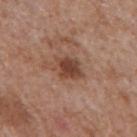{
  "biopsy_status": "not biopsied; imaged during a skin examination",
  "patient": {
    "sex": "male",
    "age_approx": 65
  },
  "automated_metrics": {
    "cielab_L": 44,
    "cielab_a": 21,
    "cielab_b": 28,
    "vs_skin_darker_L": 11.0,
    "vs_skin_contrast_norm": 8.5,
    "border_irregularity_0_10": 3.0,
    "color_variation_0_10": 3.5,
    "peripheral_color_asymmetry": 1.0
  },
  "image": {
    "source": "total-body photography crop",
    "field_of_view_mm": 15
  },
  "lighting": "white-light",
  "site": "mid back",
  "lesion_size": {
    "long_diameter_mm_approx": 3.5
  }
}